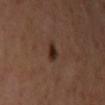| field | value |
|---|---|
| notes | catalogued during a skin exam; not biopsied |
| patient | female, in their 60s |
| imaging modality | 15 mm crop, total-body photography |
| illumination | cross-polarized illumination |
| body site | the left upper arm |
| diameter | about 2 mm |